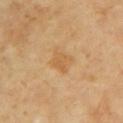{"biopsy_status": "not biopsied; imaged during a skin examination"}